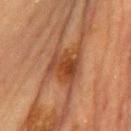<tbp_lesion>
  <biopsy_status>not biopsied; imaged during a skin examination</biopsy_status>
  <site>chest</site>
  <lesion_size>
    <long_diameter_mm_approx>4.0</long_diameter_mm_approx>
  </lesion_size>
  <lighting>cross-polarized</lighting>
  <image>
    <source>total-body photography crop</source>
    <field_of_view_mm>15</field_of_view_mm>
  </image>
  <patient>
    <sex>female</sex>
    <age_approx>60</age_approx>
  </patient>
</tbp_lesion>